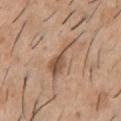Impression: Captured during whole-body skin photography for melanoma surveillance; the lesion was not biopsied. Background: A male patient in their 40s. Cropped from a whole-body photographic skin survey; the tile spans about 15 mm. This is a white-light tile. The total-body-photography lesion software estimated a mean CIELAB color near L≈54 a*≈18 b*≈30. And it measured a border-irregularity rating of about 7/10, a within-lesion color-variation index near 5.5/10, and a peripheral color-asymmetry measure near 2. And it measured an automated nevus-likeness rating near 15 out of 100 and a detector confidence of about 100 out of 100 that the crop contains a lesion. About 6 mm across. From the chest.